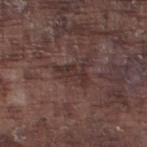Imaged during a routine full-body skin examination; the lesion was not biopsied and no histopathology is available. On the leg. Captured under white-light illumination. A region of skin cropped from a whole-body photographic capture, roughly 15 mm wide. The subject is a male aged 73–77.